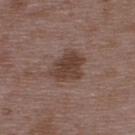Case summary:
- lighting · white-light illumination
- anatomic site · the upper back
- patient · male, approximately 50 years of age
- lesion size · ≈4 mm
- automated metrics · a shape eccentricity near 0.6 and two-axis asymmetry of about 0.25; border irregularity of about 3 on a 0–10 scale and peripheral color asymmetry of about 1
- image source · total-body-photography crop, ~15 mm field of view